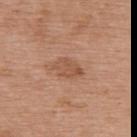biopsy_status: not biopsied; imaged during a skin examination
site: upper back
lighting: white-light
patient:
  sex: female
  age_approx: 70
image:
  source: total-body photography crop
  field_of_view_mm: 15